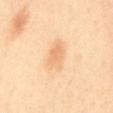Imaged during a routine full-body skin examination; the lesion was not biopsied and no histopathology is available.
A 15 mm crop from a total-body photograph taken for skin-cancer surveillance.
Imaged with cross-polarized lighting.
The lesion is located on the mid back.
A female subject aged 38 to 42.
Measured at roughly 4 mm in maximum diameter.